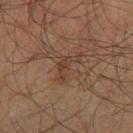Captured during whole-body skin photography for melanoma surveillance; the lesion was not biopsied. A male patient, aged 63–67. A close-up tile cropped from a whole-body skin photograph, about 15 mm across. From the right thigh. This is a cross-polarized tile.